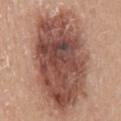Assessment: Imaged during a routine full-body skin examination; the lesion was not biopsied and no histopathology is available. Image and clinical context: The tile uses white-light illumination. Measured at roughly 13.5 mm in maximum diameter. The patient is a female about 40 years old. Cropped from a whole-body photographic skin survey; the tile spans about 15 mm. The lesion is located on the back.